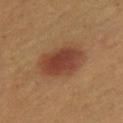Image and clinical context: A female subject aged approximately 45. A 15 mm close-up tile from a total-body photography series done for melanoma screening. From the left leg. Approximately 5 mm at its widest. Imaged with cross-polarized lighting.